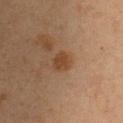The lesion was tiled from a total-body skin photograph and was not biopsied.
About 2.5 mm across.
The patient is a female aged 43 to 47.
Imaged with cross-polarized lighting.
The lesion is located on the upper back.
A lesion tile, about 15 mm wide, cut from a 3D total-body photograph.